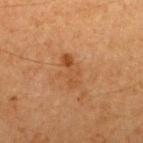Notes:
– follow-up — total-body-photography surveillance lesion; no biopsy
– imaging modality — ~15 mm tile from a whole-body skin photo
– subject — male, aged approximately 65
– site — the back